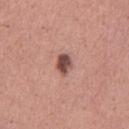follow-up=no biopsy performed (imaged during a skin exam) | acquisition=~15 mm crop, total-body skin-cancer survey | subject=male, roughly 45 years of age | size=≈2.5 mm | tile lighting=white-light illumination | image-analysis metrics=an area of roughly 4 mm² and a symmetry-axis asymmetry near 0.2; an average lesion color of about L≈49 a*≈23 b*≈24 (CIELAB), about 16 CIELAB-L* units darker than the surrounding skin, and a normalized border contrast of about 11; a border-irregularity index near 1.5/10 and a within-lesion color-variation index near 4.5/10; an automated nevus-likeness rating near 95 out of 100 and a detector confidence of about 100 out of 100 that the crop contains a lesion | location=the chest.